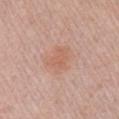  biopsy_status: not biopsied; imaged during a skin examination
  lesion_size:
    long_diameter_mm_approx: 2.5
  image:
    source: total-body photography crop
    field_of_view_mm: 15
  site: right upper arm
  patient:
    sex: male
    age_approx: 55
  automated_metrics:
    cielab_L: 60
    cielab_a: 23
    cielab_b: 30
    vs_skin_contrast_norm: 4.5
    border_irregularity_0_10: 3.0
    color_variation_0_10: 2.5
    lesion_detection_confidence_0_100: 100
  lighting: white-light